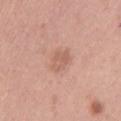| feature | finding |
|---|---|
| follow-up | catalogued during a skin exam; not biopsied |
| patient | female, approximately 55 years of age |
| anatomic site | the left thigh |
| size | ≈2.5 mm |
| image | total-body-photography crop, ~15 mm field of view |
| tile lighting | white-light illumination |
| automated metrics | a mean CIELAB color near L≈60 a*≈22 b*≈28, a lesion–skin lightness drop of about 8, and a normalized lesion–skin contrast near 5; internal color variation of about 2.5 on a 0–10 scale and radial color variation of about 1; a lesion-detection confidence of about 100/100 |